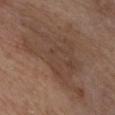Captured during whole-body skin photography for melanoma surveillance; the lesion was not biopsied.
A lesion tile, about 15 mm wide, cut from a 3D total-body photograph.
From the chest.
Longest diameter approximately 11 mm.
This is a white-light tile.
A male subject, roughly 75 years of age.